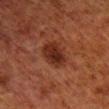Findings:
• workup: imaged on a skin check; not biopsied
• subject: male, about 80 years old
• lesion size: about 4 mm
• image source: total-body-photography crop, ~15 mm field of view
• illumination: cross-polarized illumination
• body site: the right lower leg